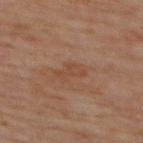Impression:
Captured during whole-body skin photography for melanoma surveillance; the lesion was not biopsied.
Image and clinical context:
A region of skin cropped from a whole-body photographic capture, roughly 15 mm wide. From the mid back. A male subject, in their 60s.